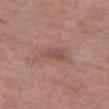This lesion was catalogued during total-body skin photography and was not selected for biopsy. Located on the head or neck. A female subject, about 70 years old. Captured under white-light illumination. This image is a 15 mm lesion crop taken from a total-body photograph.